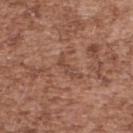A male patient aged 53–57. A 15 mm close-up tile from a total-body photography series done for melanoma screening. Longest diameter approximately 3 mm. Automated image analysis of the tile measured border irregularity of about 6.5 on a 0–10 scale and a within-lesion color-variation index near 0/10. And it measured an automated nevus-likeness rating near 0 out of 100 and a lesion-detection confidence of about 75/100. The lesion is on the upper back.